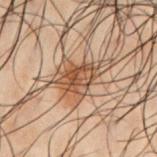notes=total-body-photography surveillance lesion; no biopsy | illumination=cross-polarized illumination | patient=male, approximately 50 years of age | anatomic site=the front of the torso | image=total-body-photography crop, ~15 mm field of view.